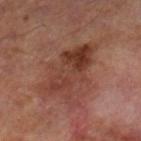Recorded during total-body skin imaging; not selected for excision or biopsy.
Longest diameter approximately 7 mm.
A male subject about 65 years old.
A 15 mm close-up tile from a total-body photography series done for melanoma screening.
The lesion is located on the right lower leg.
The lesion-visualizer software estimated a classifier nevus-likeness of about 0/100 and lesion-presence confidence of about 100/100.